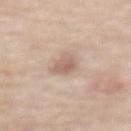notes = imaged on a skin check; not biopsied
subject = female, aged approximately 65
image = 15 mm crop, total-body photography
site = the back